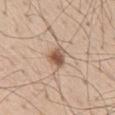Findings:
– follow-up: total-body-photography surveillance lesion; no biopsy
– automated metrics: a lesion–skin lightness drop of about 13 and a normalized border contrast of about 8.5; lesion-presence confidence of about 100/100
– patient: male, aged 58 to 62
– image source: 15 mm crop, total-body photography
– anatomic site: the mid back